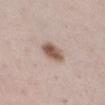biopsy status: total-body-photography surveillance lesion; no biopsy | site: the right lower leg | lesion size: about 3.5 mm | automated metrics: an area of roughly 6.5 mm², a shape eccentricity near 0.8, and two-axis asymmetry of about 0.2; a mean CIELAB color near L≈56 a*≈17 b*≈26 and about 14 CIELAB-L* units darker than the surrounding skin; border irregularity of about 2 on a 0–10 scale; a nevus-likeness score of about 95/100 | patient: female, roughly 40 years of age | imaging modality: 15 mm crop, total-body photography | tile lighting: white-light.